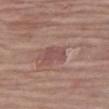Q: Was a biopsy performed?
A: no biopsy performed (imaged during a skin exam)
Q: What are the patient's age and sex?
A: female, approximately 85 years of age or older
Q: How was this image acquired?
A: ~15 mm tile from a whole-body skin photo
Q: Where on the body is the lesion?
A: the right thigh
Q: Automated lesion metrics?
A: a lesion color around L≈49 a*≈22 b*≈21 in CIELAB and a lesion–skin lightness drop of about 7; an automated nevus-likeness rating near 0 out of 100 and lesion-presence confidence of about 95/100